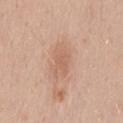Assessment: Part of a total-body skin-imaging series; this lesion was reviewed on a skin check and was not flagged for biopsy. Background: On the mid back. A 15 mm close-up extracted from a 3D total-body photography capture. The subject is a male aged 33–37.